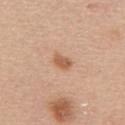Impression: Imaged during a routine full-body skin examination; the lesion was not biopsied and no histopathology is available. Clinical summary: Imaged with white-light lighting. From the mid back. A female subject in their mid-60s. This image is a 15 mm lesion crop taken from a total-body photograph. Measured at roughly 2.5 mm in maximum diameter.